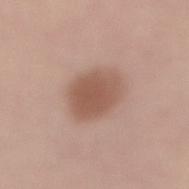Captured during whole-body skin photography for melanoma surveillance; the lesion was not biopsied. The recorded lesion diameter is about 4.5 mm. A roughly 15 mm field-of-view crop from a total-body skin photograph. Imaged with white-light lighting. The subject is a male roughly 65 years of age. The lesion is on the lower back.